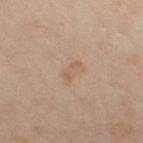| key | value |
|---|---|
| notes | catalogued during a skin exam; not biopsied |
| diameter | ~2.5 mm (longest diameter) |
| tile lighting | cross-polarized |
| image | ~15 mm crop, total-body skin-cancer survey |
| patient | female, about 50 years old |
| body site | the right thigh |